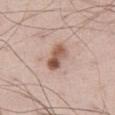lighting — white-light illumination | diameter — ≈3.5 mm | anatomic site — the left thigh | imaging modality — ~15 mm crop, total-body skin-cancer survey | patient — male, aged 58 to 62.